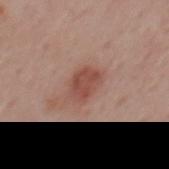Q: Was this lesion biopsied?
A: catalogued during a skin exam; not biopsied
Q: Automated lesion metrics?
A: a border-irregularity rating of about 2/10 and peripheral color asymmetry of about 1
Q: Lesion location?
A: the back
Q: Patient demographics?
A: male, aged 53–57
Q: Lesion size?
A: about 3.5 mm
Q: What kind of image is this?
A: 15 mm crop, total-body photography